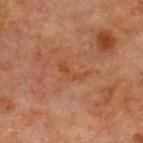  biopsy_status: not biopsied; imaged during a skin examination
  image:
    source: total-body photography crop
    field_of_view_mm: 15
  lighting: cross-polarized
  site: chest
  patient:
    sex: male
    age_approx: 65